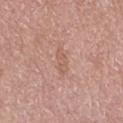{
  "biopsy_status": "not biopsied; imaged during a skin examination",
  "site": "right thigh",
  "lesion_size": {
    "long_diameter_mm_approx": 4.0
  },
  "image": {
    "source": "total-body photography crop",
    "field_of_view_mm": 15
  },
  "patient": {
    "sex": "female",
    "age_approx": 65
  }
}